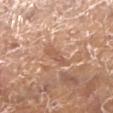The lesion was tiled from a total-body skin photograph and was not biopsied.
The recorded lesion diameter is about 3 mm.
A region of skin cropped from a whole-body photographic capture, roughly 15 mm wide.
The lesion is on the left lower leg.
A female subject, about 75 years old.